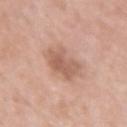Captured during whole-body skin photography for melanoma surveillance; the lesion was not biopsied.
An algorithmic analysis of the crop reported border irregularity of about 4 on a 0–10 scale. The analysis additionally found an automated nevus-likeness rating near 5 out of 100 and a lesion-detection confidence of about 100/100.
The lesion is located on the arm.
The tile uses white-light illumination.
A 15 mm close-up tile from a total-body photography series done for melanoma screening.
Approximately 4.5 mm at its widest.
A female subject, aged approximately 70.